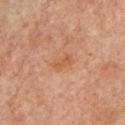Impression: No biopsy was performed on this lesion — it was imaged during a full skin examination and was not determined to be concerning. Image and clinical context: A male patient, in their mid-60s. A 15 mm close-up extracted from a 3D total-body photography capture. Automated image analysis of the tile measured a footprint of about 3.5 mm² and an eccentricity of roughly 0.85. It also reported an average lesion color of about L≈44 a*≈19 b*≈30 (CIELAB), about 5 CIELAB-L* units darker than the surrounding skin, and a lesion-to-skin contrast of about 5.5 (normalized; higher = more distinct). The software also gave a classifier nevus-likeness of about 0/100 and a detector confidence of about 100 out of 100 that the crop contains a lesion. On the upper back.